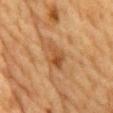| key | value |
|---|---|
| notes | imaged on a skin check; not biopsied |
| image | ~15 mm tile from a whole-body skin photo |
| subject | male, about 85 years old or older |
| body site | the chest |